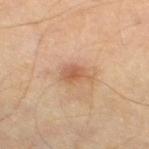notes: total-body-photography surveillance lesion; no biopsy
imaging modality: total-body-photography crop, ~15 mm field of view
body site: the right thigh
subject: roughly 55 years of age